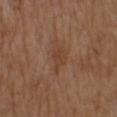No biopsy was performed on this lesion — it was imaged during a full skin examination and was not determined to be concerning. The tile uses white-light illumination. Longest diameter approximately 3 mm. This image is a 15 mm lesion crop taken from a total-body photograph. An algorithmic analysis of the crop reported an eccentricity of roughly 0.75. And it measured an average lesion color of about L≈41 a*≈19 b*≈30 (CIELAB) and a lesion–skin lightness drop of about 6. The patient is a male in their mid- to late 70s. On the upper back.